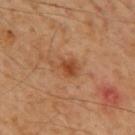A male patient aged 58 to 62. Automated tile analysis of the lesion measured a border-irregularity index near 4.5/10, internal color variation of about 2.5 on a 0–10 scale, and peripheral color asymmetry of about 0.5. It also reported an automated nevus-likeness rating near 55 out of 100. A lesion tile, about 15 mm wide, cut from a 3D total-body photograph. About 3.5 mm across. On the back.